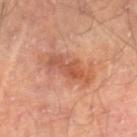notes = total-body-photography surveillance lesion; no biopsy
tile lighting = cross-polarized illumination
subject = male, approximately 70 years of age
anatomic site = the leg
lesion diameter = ≈6 mm
image = ~15 mm tile from a whole-body skin photo